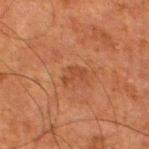Findings:
– workup — no biopsy performed (imaged during a skin exam)
– illumination — cross-polarized illumination
– imaging modality — ~15 mm tile from a whole-body skin photo
– patient — male, aged around 80
– location — the left thigh
– lesion diameter — about 2.5 mm